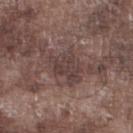This lesion was catalogued during total-body skin photography and was not selected for biopsy. On the right lower leg. The subject is a male in their mid- to late 70s. A close-up tile cropped from a whole-body skin photograph, about 15 mm across. Imaged with white-light lighting.